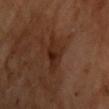Recorded during total-body skin imaging; not selected for excision or biopsy.
A male patient about 65 years old.
A lesion tile, about 15 mm wide, cut from a 3D total-body photograph.
From the chest.